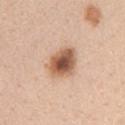| feature | finding |
|---|---|
| follow-up | total-body-photography surveillance lesion; no biopsy |
| image-analysis metrics | a mean CIELAB color near L≈58 a*≈20 b*≈32, a lesion–skin lightness drop of about 17, and a normalized lesion–skin contrast near 10.5; a color-variation rating of about 7/10 and peripheral color asymmetry of about 1.5; a nevus-likeness score of about 95/100 and lesion-presence confidence of about 100/100 |
| diameter | ~4 mm (longest diameter) |
| patient | female, in their 40s |
| site | the left upper arm |
| image | total-body-photography crop, ~15 mm field of view |
| tile lighting | white-light illumination |